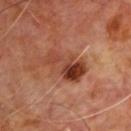Findings:
- follow-up — no biopsy performed (imaged during a skin exam)
- lighting — cross-polarized
- patient — male, about 60 years old
- image — ~15 mm tile from a whole-body skin photo
- lesion size — ~5.5 mm (longest diameter)
- site — the upper back